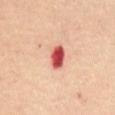{"biopsy_status": "not biopsied; imaged during a skin examination", "image": {"source": "total-body photography crop", "field_of_view_mm": 15}, "site": "front of the torso", "patient": {"sex": "female", "age_approx": 45}, "automated_metrics": {"cielab_L": 54, "cielab_a": 39, "cielab_b": 30, "vs_skin_darker_L": 21.0, "vs_skin_contrast_norm": 13.0, "border_irregularity_0_10": 2.0, "color_variation_0_10": 4.0, "peripheral_color_asymmetry": 1.0, "nevus_likeness_0_100": 0, "lesion_detection_confidence_0_100": 100}, "lesion_size": {"long_diameter_mm_approx": 3.0}, "lighting": "cross-polarized"}